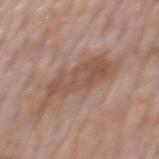biopsy status: catalogued during a skin exam; not biopsied
diameter: ~8.5 mm (longest diameter)
body site: the back
imaging modality: ~15 mm crop, total-body skin-cancer survey
patient: male, aged 73–77
image-analysis metrics: an area of roughly 20 mm², an outline eccentricity of about 0.9 (0 = round, 1 = elongated), and two-axis asymmetry of about 0.45; an average lesion color of about L≈52 a*≈18 b*≈26 (CIELAB), roughly 8 lightness units darker than nearby skin, and a normalized border contrast of about 6; border irregularity of about 7 on a 0–10 scale, internal color variation of about 3 on a 0–10 scale, and peripheral color asymmetry of about 1